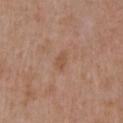The lesion was tiled from a total-body skin photograph and was not biopsied. From the right upper arm. Cropped from a whole-body photographic skin survey; the tile spans about 15 mm. Captured under white-light illumination. A male patient, aged 48 to 52.